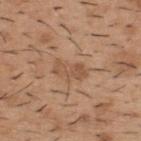Case summary:
* workup: catalogued during a skin exam; not biopsied
* lighting: white-light illumination
* lesion diameter: ≈4 mm
* automated lesion analysis: an area of roughly 5 mm², an outline eccentricity of about 0.9 (0 = round, 1 = elongated), and a symmetry-axis asymmetry near 0.35; a mean CIELAB color near L≈52 a*≈20 b*≈32, about 8 CIELAB-L* units darker than the surrounding skin, and a normalized border contrast of about 6; a detector confidence of about 100 out of 100 that the crop contains a lesion
* image source: total-body-photography crop, ~15 mm field of view
* subject: male, aged approximately 40
* anatomic site: the upper back